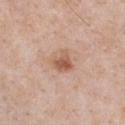No biopsy was performed on this lesion — it was imaged during a full skin examination and was not determined to be concerning.
The lesion's longest dimension is about 2.5 mm.
Automated tile analysis of the lesion measured a lesion area of about 5.5 mm², an outline eccentricity of about 0.35 (0 = round, 1 = elongated), and a shape-asymmetry score of about 0.25 (0 = symmetric). The software also gave an average lesion color of about L≈57 a*≈21 b*≈30 (CIELAB), a lesion–skin lightness drop of about 11, and a lesion-to-skin contrast of about 7.5 (normalized; higher = more distinct). It also reported a classifier nevus-likeness of about 65/100 and lesion-presence confidence of about 100/100.
The lesion is located on the front of the torso.
Cropped from a whole-body photographic skin survey; the tile spans about 15 mm.
A male subject aged approximately 50.
Imaged with white-light lighting.